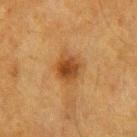* follow-up: catalogued during a skin exam; not biopsied
* acquisition: ~15 mm crop, total-body skin-cancer survey
* body site: the right forearm
* lesion diameter: about 3.5 mm
* tile lighting: cross-polarized illumination
* subject: female, aged 53 to 57